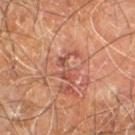No biopsy was performed on this lesion — it was imaged during a full skin examination and was not determined to be concerning. Automated tile analysis of the lesion measured a mean CIELAB color near L≈50 a*≈27 b*≈29 and roughly 8 lightness units darker than nearby skin. The software also gave an automated nevus-likeness rating near 0 out of 100 and a detector confidence of about 100 out of 100 that the crop contains a lesion. Imaged with cross-polarized lighting. Cropped from a total-body skin-imaging series; the visible field is about 15 mm. Measured at roughly 4 mm in maximum diameter. The lesion is located on the right leg. The patient is a male aged 58 to 62.